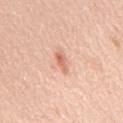follow-up: catalogued during a skin exam; not biopsied | acquisition: 15 mm crop, total-body photography | patient: male, approximately 65 years of age | body site: the upper back.